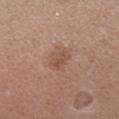Clinical impression:
Recorded during total-body skin imaging; not selected for excision or biopsy.
Context:
An algorithmic analysis of the crop reported a shape eccentricity near 0.55 and a shape-asymmetry score of about 0.35 (0 = symmetric). And it measured about 7 CIELAB-L* units darker than the surrounding skin and a lesion-to-skin contrast of about 6 (normalized; higher = more distinct). On the left forearm. About 3 mm across. A region of skin cropped from a whole-body photographic capture, roughly 15 mm wide. A female subject aged around 30. This is a white-light tile.